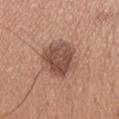<tbp_lesion>
<biopsy_status>not biopsied; imaged during a skin examination</biopsy_status>
<patient>
  <sex>male</sex>
  <age_approx>30</age_approx>
</patient>
<lesion_size>
  <long_diameter_mm_approx>5.5</long_diameter_mm_approx>
</lesion_size>
<automated_metrics>
  <border_irregularity_0_10>2.5</border_irregularity_0_10>
  <color_variation_0_10>4.5</color_variation_0_10>
  <peripheral_color_asymmetry>1.5</peripheral_color_asymmetry>
</automated_metrics>
<site>head or neck</site>
<image>
  <source>total-body photography crop</source>
  <field_of_view_mm>15</field_of_view_mm>
</image>
<lighting>white-light</lighting>
</tbp_lesion>